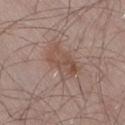The lesion was photographed on a routine skin check and not biopsied; there is no pathology result.